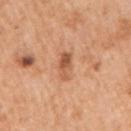Case summary:
- lesion diameter — ≈3.5 mm
- image — ~15 mm tile from a whole-body skin photo
- patient — female, aged 53 to 57
- body site — the right upper arm